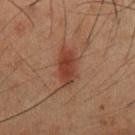Captured during whole-body skin photography for melanoma surveillance; the lesion was not biopsied. The tile uses cross-polarized illumination. This image is a 15 mm lesion crop taken from a total-body photograph. A male subject in their 50s. Located on the chest. Automated image analysis of the tile measured an average lesion color of about L≈30 a*≈18 b*≈23 (CIELAB), a lesion–skin lightness drop of about 7, and a lesion-to-skin contrast of about 7.5 (normalized; higher = more distinct). The analysis additionally found a nevus-likeness score of about 90/100 and a lesion-detection confidence of about 100/100.